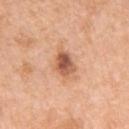This lesion was catalogued during total-body skin photography and was not selected for biopsy.
Longest diameter approximately 3.5 mm.
The patient is a female approximately 55 years of age.
This image is a 15 mm lesion crop taken from a total-body photograph.
Imaged with white-light lighting.
The lesion is located on the arm.
Automated image analysis of the tile measured a shape eccentricity near 0.65. It also reported a lesion color around L≈57 a*≈25 b*≈34 in CIELAB and roughly 15 lightness units darker than nearby skin. It also reported a border-irregularity rating of about 1.5/10 and a peripheral color-asymmetry measure near 2.5.